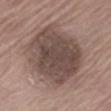Recorded during total-body skin imaging; not selected for excision or biopsy.
The lesion-visualizer software estimated a lesion color around L≈46 a*≈15 b*≈21 in CIELAB, about 12 CIELAB-L* units darker than the surrounding skin, and a normalized lesion–skin contrast near 9. It also reported a border-irregularity index near 2/10, internal color variation of about 4.5 on a 0–10 scale, and radial color variation of about 1.5.
This image is a 15 mm lesion crop taken from a total-body photograph.
The tile uses white-light illumination.
The lesion is on the right thigh.
A male subject roughly 65 years of age.
Measured at roughly 7.5 mm in maximum diameter.